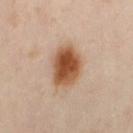notes: total-body-photography surveillance lesion; no biopsy
site: the right thigh
patient: female, in their 40s
image source: 15 mm crop, total-body photography